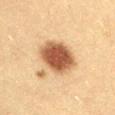The lesion was photographed on a routine skin check and not biopsied; there is no pathology result.
The recorded lesion diameter is about 5 mm.
The lesion is on the abdomen.
A female patient, aged 28–32.
A close-up tile cropped from a whole-body skin photograph, about 15 mm across.